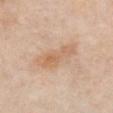Q: Is there a histopathology result?
A: no biopsy performed (imaged during a skin exam)
Q: How was this image acquired?
A: ~15 mm tile from a whole-body skin photo
Q: How was the tile lit?
A: white-light illumination
Q: What is the lesion's diameter?
A: about 6 mm
Q: Lesion location?
A: the chest
Q: Who is the patient?
A: female, in their mid- to late 60s